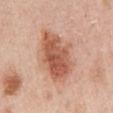follow-up: catalogued during a skin exam; not biopsied | patient: female, approximately 50 years of age | illumination: white-light illumination | automated metrics: an area of roughly 23 mm² and a shape-asymmetry score of about 0.2 (0 = symmetric); a mean CIELAB color near L≈58 a*≈25 b*≈32, roughly 13 lightness units darker than nearby skin, and a normalized lesion–skin contrast near 9; a classifier nevus-likeness of about 95/100 and a lesion-detection confidence of about 100/100 | anatomic site: the left upper arm | acquisition: 15 mm crop, total-body photography.